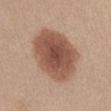workup — no biopsy performed (imaged during a skin exam) | image — 15 mm crop, total-body photography | patient — female, aged 43 to 47 | location — the front of the torso.